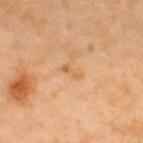<tbp_lesion>
  <biopsy_status>not biopsied; imaged during a skin examination</biopsy_status>
  <image>
    <source>total-body photography crop</source>
    <field_of_view_mm>15</field_of_view_mm>
  </image>
  <automated_metrics>
    <area_mm2_approx>2.5</area_mm2_approx>
    <eccentricity>0.9</eccentricity>
    <shape_asymmetry>0.4</shape_asymmetry>
    <border_irregularity_0_10>4.5</border_irregularity_0_10>
    <color_variation_0_10>0.0</color_variation_0_10>
    <peripheral_color_asymmetry>0.0</peripheral_color_asymmetry>
  </automated_metrics>
  <site>back</site>
  <lesion_size>
    <long_diameter_mm_approx>2.5</long_diameter_mm_approx>
  </lesion_size>
  <lighting>cross-polarized</lighting>
  <patient>
    <sex>male</sex>
    <age_approx>40</age_approx>
  </patient>
</tbp_lesion>